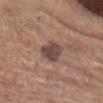Q: Was this lesion biopsied?
A: catalogued during a skin exam; not biopsied
Q: What is the lesion's diameter?
A: ~3.5 mm (longest diameter)
Q: Illumination type?
A: white-light
Q: What is the imaging modality?
A: ~15 mm tile from a whole-body skin photo
Q: What are the patient's age and sex?
A: male, aged 63–67
Q: Lesion location?
A: the head or neck